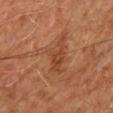{
  "biopsy_status": "not biopsied; imaged during a skin examination",
  "image": {
    "source": "total-body photography crop",
    "field_of_view_mm": 15
  },
  "site": "chest",
  "lighting": "cross-polarized",
  "patient": {
    "sex": "male",
    "age_approx": 60
  }
}